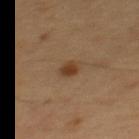workup=catalogued during a skin exam; not biopsied | location=the upper back | subject=male, aged around 60 | illumination=cross-polarized illumination | size=≈2 mm | acquisition=~15 mm tile from a whole-body skin photo.